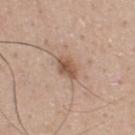Impression: This lesion was catalogued during total-body skin photography and was not selected for biopsy. Acquisition and patient details: The lesion is located on the upper back. A male subject, roughly 30 years of age. A 15 mm crop from a total-body photograph taken for skin-cancer surveillance.